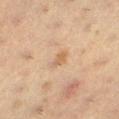Imaged during a routine full-body skin examination; the lesion was not biopsied and no histopathology is available. Captured under cross-polarized illumination. Longest diameter approximately 2.5 mm. The lesion is located on the left lower leg. The subject is a female aged 38 to 42. A 15 mm crop from a total-body photograph taken for skin-cancer surveillance.